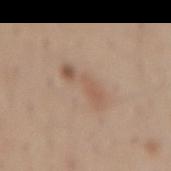- notes · no biopsy performed (imaged during a skin exam)
- lighting · white-light
- subject · male, roughly 55 years of age
- acquisition · ~15 mm crop, total-body skin-cancer survey
- diameter · ≈5 mm
- automated metrics · an eccentricity of roughly 0.9; a classifier nevus-likeness of about 15/100
- site · the lower back